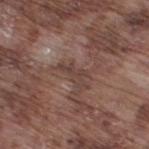– follow-up: catalogued during a skin exam; not biopsied